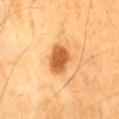<case>
  <image>
    <source>total-body photography crop</source>
    <field_of_view_mm>15</field_of_view_mm>
  </image>
  <site>leg</site>
  <patient>
    <sex>male</sex>
    <age_approx>60</age_approx>
  </patient>
</case>